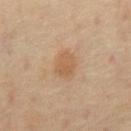Part of a total-body skin-imaging series; this lesion was reviewed on a skin check and was not flagged for biopsy.
This is a cross-polarized tile.
About 3 mm across.
A male subject aged approximately 65.
On the front of the torso.
A roughly 15 mm field-of-view crop from a total-body skin photograph.